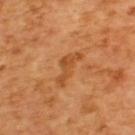Assessment: Recorded during total-body skin imaging; not selected for excision or biopsy. Acquisition and patient details: This image is a 15 mm lesion crop taken from a total-body photograph. A male patient roughly 65 years of age. The lesion is on the back.